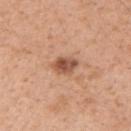Q: Was a biopsy performed?
A: no biopsy performed (imaged during a skin exam)
Q: Automated lesion metrics?
A: a lesion area of about 5.5 mm², a shape eccentricity near 0.55, and two-axis asymmetry of about 0.15
Q: Illumination type?
A: white-light
Q: What are the patient's age and sex?
A: male, aged around 30
Q: What is the anatomic site?
A: the right upper arm
Q: What kind of image is this?
A: ~15 mm tile from a whole-body skin photo
Q: What is the lesion's diameter?
A: ~3 mm (longest diameter)